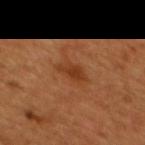The lesion was photographed on a routine skin check and not biopsied; there is no pathology result. Automated tile analysis of the lesion measured a footprint of about 5 mm², an outline eccentricity of about 0.8 (0 = round, 1 = elongated), and two-axis asymmetry of about 0.3. And it measured a border-irregularity rating of about 3/10, internal color variation of about 2.5 on a 0–10 scale, and peripheral color asymmetry of about 0.5. It also reported a nevus-likeness score of about 25/100 and a detector confidence of about 100 out of 100 that the crop contains a lesion. A roughly 15 mm field-of-view crop from a total-body skin photograph. About 3 mm across. The patient is a male aged approximately 45. Imaged with cross-polarized lighting. On the mid back.